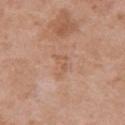| key | value |
|---|---|
| biopsy status | no biopsy performed (imaged during a skin exam) |